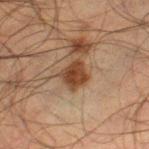Q: Was this lesion biopsied?
A: total-body-photography surveillance lesion; no biopsy
Q: How large is the lesion?
A: ~3 mm (longest diameter)
Q: What are the patient's age and sex?
A: male, approximately 50 years of age
Q: What lighting was used for the tile?
A: cross-polarized
Q: Where on the body is the lesion?
A: the left thigh
Q: What is the imaging modality?
A: ~15 mm tile from a whole-body skin photo